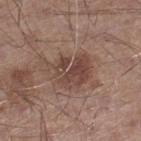Clinical impression:
No biopsy was performed on this lesion — it was imaged during a full skin examination and was not determined to be concerning.
Context:
From the right lower leg. A male subject approximately 55 years of age. The recorded lesion diameter is about 5.5 mm. Captured under white-light illumination. Automated tile analysis of the lesion measured a border-irregularity index near 5.5/10, a color-variation rating of about 4/10, and radial color variation of about 1.5. And it measured a nevus-likeness score of about 35/100. A 15 mm crop from a total-body photograph taken for skin-cancer surveillance.